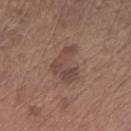workup = total-body-photography surveillance lesion; no biopsy
size = about 5 mm
acquisition = total-body-photography crop, ~15 mm field of view
automated lesion analysis = border irregularity of about 5.5 on a 0–10 scale, a within-lesion color-variation index near 4/10, and a peripheral color-asymmetry measure near 1.5; an automated nevus-likeness rating near 10 out of 100
body site = the right forearm
lighting = white-light
patient = male, in their mid-60s